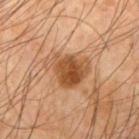No biopsy was performed on this lesion — it was imaged during a full skin examination and was not determined to be concerning.